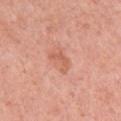No biopsy was performed on this lesion — it was imaged during a full skin examination and was not determined to be concerning. A female subject, in their mid-30s. The lesion is on the left upper arm. A lesion tile, about 15 mm wide, cut from a 3D total-body photograph. Imaged with white-light lighting.